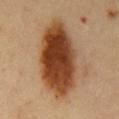The lesion was tiled from a total-body skin photograph and was not biopsied. A male subject about 50 years old. The lesion is located on the chest. A 15 mm crop from a total-body photograph taken for skin-cancer surveillance.